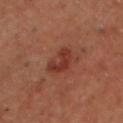Assessment:
Part of a total-body skin-imaging series; this lesion was reviewed on a skin check and was not flagged for biopsy.
Background:
An algorithmic analysis of the crop reported an area of roughly 6.5 mm², an eccentricity of roughly 0.8, and a shape-asymmetry score of about 0.4 (0 = symmetric). And it measured a lesion color around L≈26 a*≈21 b*≈23 in CIELAB. The software also gave peripheral color asymmetry of about 0.5. A 15 mm close-up tile from a total-body photography series done for melanoma screening. This is a cross-polarized tile. The lesion's longest dimension is about 3.5 mm. Located on the chest. The patient is a male aged approximately 50.